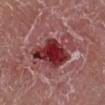Clinical impression:
The lesion was photographed on a routine skin check and not biopsied; there is no pathology result.
Clinical summary:
Cropped from a whole-body photographic skin survey; the tile spans about 15 mm. From the right lower leg. Imaged with white-light lighting. A male patient in their mid- to late 60s. Approximately 8 mm at its widest.